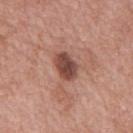notes: no biopsy performed (imaged during a skin exam) | automated metrics: a lesion area of about 7.5 mm²; an average lesion color of about L≈46 a*≈23 b*≈25 (CIELAB) and a normalized lesion–skin contrast near 10 | image: ~15 mm tile from a whole-body skin photo | subject: male, aged 48 to 52 | site: the mid back.